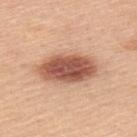Q: Is there a histopathology result?
A: total-body-photography surveillance lesion; no biopsy
Q: Who is the patient?
A: male, aged 48 to 52
Q: What lighting was used for the tile?
A: white-light
Q: Lesion location?
A: the upper back
Q: How large is the lesion?
A: ~7 mm (longest diameter)
Q: Automated lesion metrics?
A: a mean CIELAB color near L≈56 a*≈25 b*≈31, about 18 CIELAB-L* units darker than the surrounding skin, and a normalized lesion–skin contrast near 11; a color-variation rating of about 5/10
Q: What kind of image is this?
A: 15 mm crop, total-body photography